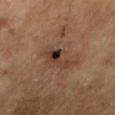This lesion was catalogued during total-body skin photography and was not selected for biopsy.